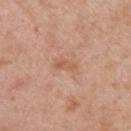biopsy status: total-body-photography surveillance lesion; no biopsy | illumination: white-light | site: the right upper arm | patient: female, about 40 years old | lesion diameter: ~3 mm (longest diameter) | imaging modality: 15 mm crop, total-body photography.